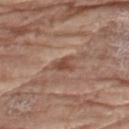  image:
    source: total-body photography crop
    field_of_view_mm: 15
  lesion_size:
    long_diameter_mm_approx: 3.0
  lighting: white-light
  patient:
    sex: female
    age_approx: 75
  site: left thigh